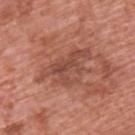| feature | finding |
|---|---|
| biopsy status | imaged on a skin check; not biopsied |
| illumination | white-light |
| diameter | ~6.5 mm (longest diameter) |
| image-analysis metrics | an average lesion color of about L≈48 a*≈25 b*≈28 (CIELAB), roughly 9 lightness units darker than nearby skin, and a normalized border contrast of about 6.5 |
| image | ~15 mm tile from a whole-body skin photo |
| patient | male, about 70 years old |
| body site | the back |